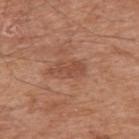Captured during whole-body skin photography for melanoma surveillance; the lesion was not biopsied. On the right upper arm. A 15 mm close-up tile from a total-body photography series done for melanoma screening. The patient is a male roughly 65 years of age.